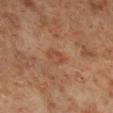| field | value |
|---|---|
| follow-up | imaged on a skin check; not biopsied |
| body site | the right lower leg |
| acquisition | ~15 mm crop, total-body skin-cancer survey |
| subject | male, in their 60s |
| tile lighting | cross-polarized |
| image-analysis metrics | a lesion area of about 4 mm² and a shape-asymmetry score of about 0.25 (0 = symmetric); a mean CIELAB color near L≈44 a*≈22 b*≈30 and a normalized lesion–skin contrast near 5; a border-irregularity rating of about 2/10, a within-lesion color-variation index near 3/10, and peripheral color asymmetry of about 1.5 |
| diameter | about 2.5 mm |